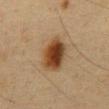Part of a total-body skin-imaging series; this lesion was reviewed on a skin check and was not flagged for biopsy. This is a cross-polarized tile. The lesion is on the abdomen. A male subject, roughly 60 years of age. Automated tile analysis of the lesion measured an area of roughly 12 mm², an eccentricity of roughly 0.7, and a symmetry-axis asymmetry near 0.15. It also reported a lesion color around L≈32 a*≈16 b*≈28 in CIELAB, about 13 CIELAB-L* units darker than the surrounding skin, and a lesion-to-skin contrast of about 12 (normalized; higher = more distinct). And it measured a border-irregularity rating of about 1.5/10, a color-variation rating of about 6/10, and peripheral color asymmetry of about 1.5. The recorded lesion diameter is about 5 mm. Cropped from a whole-body photographic skin survey; the tile spans about 15 mm.